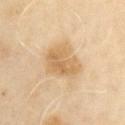Recorded during total-body skin imaging; not selected for excision or biopsy.
A male subject about 65 years old.
The lesion is on the right upper arm.
Imaged with cross-polarized lighting.
Approximately 4.5 mm at its widest.
A 15 mm close-up extracted from a 3D total-body photography capture.